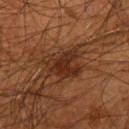Findings:
* workup — total-body-photography surveillance lesion; no biopsy
* imaging modality — ~15 mm crop, total-body skin-cancer survey
* lighting — cross-polarized
* anatomic site — the left upper arm
* automated lesion analysis — a footprint of about 12 mm² and a shape-asymmetry score of about 0.3 (0 = symmetric); a nevus-likeness score of about 55/100 and lesion-presence confidence of about 95/100
* patient — male, aged 43 to 47
* lesion diameter — ≈5 mm